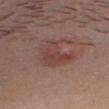notes = catalogued during a skin exam; not biopsied
lesion diameter = ~4.5 mm (longest diameter)
anatomic site = the head or neck
acquisition = ~15 mm crop, total-body skin-cancer survey
patient = male, aged around 30
illumination = white-light illumination